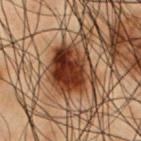Impression: Recorded during total-body skin imaging; not selected for excision or biopsy.